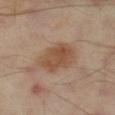Q: Is there a histopathology result?
A: total-body-photography surveillance lesion; no biopsy
Q: What did automated image analysis measure?
A: border irregularity of about 2 on a 0–10 scale, a color-variation rating of about 3.5/10, and radial color variation of about 1
Q: Illumination type?
A: cross-polarized illumination
Q: How was this image acquired?
A: 15 mm crop, total-body photography
Q: What are the patient's age and sex?
A: male, in their mid- to late 60s
Q: Lesion location?
A: the right lower leg
Q: How large is the lesion?
A: ≈5 mm